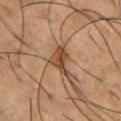Impression: Part of a total-body skin-imaging series; this lesion was reviewed on a skin check and was not flagged for biopsy. Background: About 4.5 mm across. A close-up tile cropped from a whole-body skin photograph, about 15 mm across. From the chest. A male subject, in their mid- to late 50s.